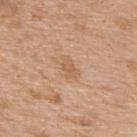Imaged during a routine full-body skin examination; the lesion was not biopsied and no histopathology is available. A male patient in their mid-60s. On the upper back. A lesion tile, about 15 mm wide, cut from a 3D total-body photograph.